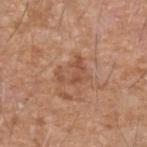<lesion>
  <biopsy_status>not biopsied; imaged during a skin examination</biopsy_status>
  <site>left forearm</site>
  <patient>
    <sex>male</sex>
    <age_approx>75</age_approx>
  </patient>
  <automated_metrics>
    <color_variation_0_10>1.5</color_variation_0_10>
    <peripheral_color_asymmetry>0.5</peripheral_color_asymmetry>
    <nevus_likeness_0_100>5</nevus_likeness_0_100>
    <lesion_detection_confidence_0_100>100</lesion_detection_confidence_0_100>
  </automated_metrics>
  <lesion_size>
    <long_diameter_mm_approx>3.5</long_diameter_mm_approx>
  </lesion_size>
  <image>
    <source>total-body photography crop</source>
    <field_of_view_mm>15</field_of_view_mm>
  </image>
</lesion>